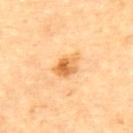follow-up: no biopsy performed (imaged during a skin exam); patient: male, approximately 85 years of age or older; tile lighting: cross-polarized illumination; size: about 3.5 mm; imaging modality: 15 mm crop, total-body photography; location: the upper back.